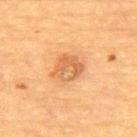Case summary:
• imaging modality · ~15 mm tile from a whole-body skin photo
• lighting · cross-polarized illumination
• subject · female, aged around 70
• site · the upper back